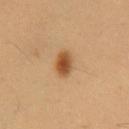The lesion was tiled from a total-body skin photograph and was not biopsied.
A 15 mm close-up extracted from a 3D total-body photography capture.
The lesion is located on the abdomen.
A male subject, in their mid- to late 50s.
An algorithmic analysis of the crop reported a footprint of about 5 mm² and an outline eccentricity of about 0.75 (0 = round, 1 = elongated). It also reported a border-irregularity index near 1.5/10, a within-lesion color-variation index near 4.5/10, and a peripheral color-asymmetry measure near 1.5. The analysis additionally found a classifier nevus-likeness of about 100/100 and a lesion-detection confidence of about 100/100.